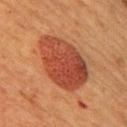Part of a total-body skin-imaging series; this lesion was reviewed on a skin check and was not flagged for biopsy. From the right upper arm. Captured under cross-polarized illumination. A female patient aged approximately 50. Measured at roughly 7.5 mm in maximum diameter. The total-body-photography lesion software estimated a footprint of about 27 mm², an eccentricity of roughly 0.75, and a shape-asymmetry score of about 0.15 (0 = symmetric). It also reported an average lesion color of about L≈38 a*≈26 b*≈30 (CIELAB), a lesion–skin lightness drop of about 11, and a normalized lesion–skin contrast near 9. And it measured a border-irregularity index near 2/10 and a peripheral color-asymmetry measure near 1.5. The software also gave a classifier nevus-likeness of about 100/100 and a lesion-detection confidence of about 100/100. A roughly 15 mm field-of-view crop from a total-body skin photograph.